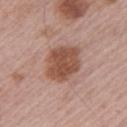  biopsy_status: not biopsied; imaged during a skin examination
  image:
    source: total-body photography crop
    field_of_view_mm: 15
  lesion_size:
    long_diameter_mm_approx: 5.5
  site: arm
  lighting: white-light
  patient:
    sex: male
    age_approx: 65
  automated_metrics:
    area_mm2_approx: 15.0
    shape_asymmetry: 0.15
    cielab_L: 51
    cielab_a: 22
    cielab_b: 28
    vs_skin_darker_L: 12.0
    vs_skin_contrast_norm: 9.0